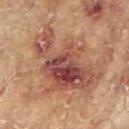biopsy_status: not biopsied; imaged during a skin examination
site: left arm
lesion_size:
  long_diameter_mm_approx: 9.0
image:
  source: total-body photography crop
  field_of_view_mm: 15
lighting: cross-polarized
automated_metrics:
  cielab_L: 44
  cielab_a: 23
  cielab_b: 25
  vs_skin_darker_L: 11.0
  vs_skin_contrast_norm: 9.5
  border_irregularity_0_10: 8.0
  peripheral_color_asymmetry: 2.5
  nevus_likeness_0_100: 0
  lesion_detection_confidence_0_100: 100
patient:
  sex: female
  age_approx: 80